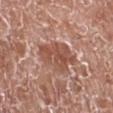Q: Was a biopsy performed?
A: imaged on a skin check; not biopsied
Q: Who is the patient?
A: male, aged 73–77
Q: What lighting was used for the tile?
A: white-light
Q: Where on the body is the lesion?
A: the leg
Q: What is the imaging modality?
A: 15 mm crop, total-body photography